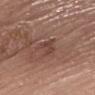The lesion was photographed on a routine skin check and not biopsied; there is no pathology result. A female subject roughly 85 years of age or older. From the upper back. A 15 mm crop from a total-body photograph taken for skin-cancer surveillance.